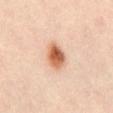The lesion was photographed on a routine skin check and not biopsied; there is no pathology result. The patient is a male about 65 years old. This is a cross-polarized tile. Automated image analysis of the tile measured a shape eccentricity near 0.7 and a shape-asymmetry score of about 0.15 (0 = symmetric). It also reported a classifier nevus-likeness of about 100/100. A region of skin cropped from a whole-body photographic capture, roughly 15 mm wide. The lesion is on the abdomen.